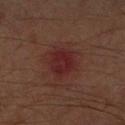{"lighting": "cross-polarized", "image": {"source": "total-body photography crop", "field_of_view_mm": 15}, "automated_metrics": {"area_mm2_approx": 9.5, "eccentricity": 0.35, "shape_asymmetry": 0.2, "vs_skin_darker_L": 6.0, "vs_skin_contrast_norm": 7.0, "border_irregularity_0_10": 2.0, "color_variation_0_10": 3.0, "peripheral_color_asymmetry": 1.0}, "patient": {"sex": "male", "age_approx": 60}, "site": "right forearm"}